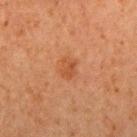Q: Was this lesion biopsied?
A: no biopsy performed (imaged during a skin exam)
Q: Who is the patient?
A: female, aged 58 to 62
Q: Lesion size?
A: about 2.5 mm
Q: What is the imaging modality?
A: ~15 mm crop, total-body skin-cancer survey
Q: How was the tile lit?
A: cross-polarized
Q: Lesion location?
A: the right upper arm